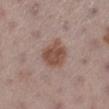Impression: The lesion was tiled from a total-body skin photograph and was not biopsied. Context: An algorithmic analysis of the crop reported an area of roughly 10 mm² and an outline eccentricity of about 0.55 (0 = round, 1 = elongated). And it measured an average lesion color of about L≈49 a*≈19 b*≈26 (CIELAB), roughly 11 lightness units darker than nearby skin, and a normalized border contrast of about 9. Cropped from a whole-body photographic skin survey; the tile spans about 15 mm. On the left lower leg. The tile uses white-light illumination. A female patient approximately 55 years of age.